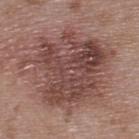Assessment: No biopsy was performed on this lesion — it was imaged during a full skin examination and was not determined to be concerning. Context: Automated tile analysis of the lesion measured border irregularity of about 6.5 on a 0–10 scale and peripheral color asymmetry of about 2.5. The tile uses white-light illumination. A 15 mm close-up extracted from a 3D total-body photography capture. The lesion's longest dimension is about 10.5 mm. The lesion is on the upper back. A male subject, in their 50s.